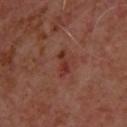Imaged during a routine full-body skin examination; the lesion was not biopsied and no histopathology is available.
This image is a 15 mm lesion crop taken from a total-body photograph.
The lesion is located on the upper back.
The patient is a male aged 48 to 52.
Imaged with cross-polarized lighting.
Approximately 3 mm at its widest.